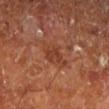Impression:
Part of a total-body skin-imaging series; this lesion was reviewed on a skin check and was not flagged for biopsy.
Context:
The lesion is on the left lower leg. The lesion's longest dimension is about 4 mm. The lesion-visualizer software estimated an outline eccentricity of about 0.75 (0 = round, 1 = elongated) and two-axis asymmetry of about 0.25. The analysis additionally found a mean CIELAB color near L≈38 a*≈25 b*≈30, a lesion–skin lightness drop of about 7, and a lesion-to-skin contrast of about 6 (normalized; higher = more distinct). It also reported a color-variation rating of about 2.5/10 and a peripheral color-asymmetry measure near 1. A male patient, in their mid- to late 60s. A roughly 15 mm field-of-view crop from a total-body skin photograph.